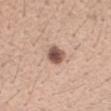The lesion was photographed on a routine skin check and not biopsied; there is no pathology result.
From the left forearm.
Captured under white-light illumination.
The lesion's longest dimension is about 3 mm.
The subject is a female aged 43 to 47.
Cropped from a whole-body photographic skin survey; the tile spans about 15 mm.